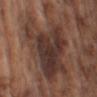Clinical impression: Captured during whole-body skin photography for melanoma surveillance; the lesion was not biopsied. Image and clinical context: A lesion tile, about 15 mm wide, cut from a 3D total-body photograph. The lesion is located on the left upper arm. A male patient, aged approximately 75.